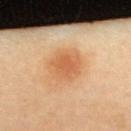This lesion was catalogued during total-body skin photography and was not selected for biopsy. A 15 mm crop from a total-body photograph taken for skin-cancer surveillance. The subject is a female aged approximately 40. Longest diameter approximately 4 mm. From the upper back. Automated image analysis of the tile measured a footprint of about 12 mm², an eccentricity of roughly 0.3, and two-axis asymmetry of about 0.1. The analysis additionally found an average lesion color of about L≈64 a*≈25 b*≈41 (CIELAB), roughly 10 lightness units darker than nearby skin, and a normalized border contrast of about 6.5. It also reported border irregularity of about 1.5 on a 0–10 scale, a color-variation rating of about 3.5/10, and radial color variation of about 1. The software also gave a lesion-detection confidence of about 100/100.